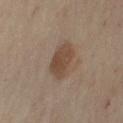Assessment:
Imaged during a routine full-body skin examination; the lesion was not biopsied and no histopathology is available.
Background:
Captured under cross-polarized illumination. A female patient in their 50s. The lesion is on the left upper arm. A lesion tile, about 15 mm wide, cut from a 3D total-body photograph. Automated image analysis of the tile measured a classifier nevus-likeness of about 75/100 and lesion-presence confidence of about 100/100. About 4 mm across.